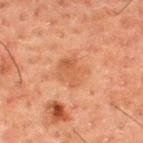Clinical impression:
This lesion was catalogued during total-body skin photography and was not selected for biopsy.
Image and clinical context:
Located on the upper back. A male patient, roughly 50 years of age. This image is a 15 mm lesion crop taken from a total-body photograph.